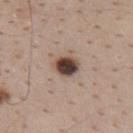| feature | finding |
|---|---|
| follow-up | imaged on a skin check; not biopsied |
| lesion diameter | ≈3 mm |
| subject | male, aged 38–42 |
| acquisition | total-body-photography crop, ~15 mm field of view |
| anatomic site | the back |
| lighting | white-light illumination |